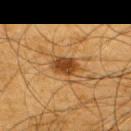<case>
<biopsy_status>not biopsied; imaged during a skin examination</biopsy_status>
<image>
  <source>total-body photography crop</source>
  <field_of_view_mm>15</field_of_view_mm>
</image>
<site>right upper arm</site>
<lesion_size>
  <long_diameter_mm_approx>3.5</long_diameter_mm_approx>
</lesion_size>
<patient>
  <sex>male</sex>
  <age_approx>65</age_approx>
</patient>
<lighting>cross-polarized</lighting>
<automated_metrics>
  <cielab_L>36</cielab_L>
  <cielab_a>19</cielab_a>
  <cielab_b>34</cielab_b>
  <vs_skin_darker_L>12.0</vs_skin_darker_L>
</automated_metrics>
</case>